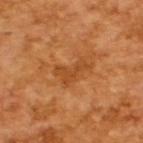image:
  source: total-body photography crop
  field_of_view_mm: 15
patient:
  sex: male
  age_approx: 65